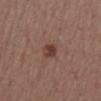| feature | finding |
|---|---|
| follow-up | total-body-photography surveillance lesion; no biopsy |